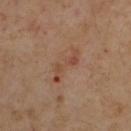lesion size=~5 mm (longest diameter) | automated lesion analysis=a lesion area of about 5.5 mm², an eccentricity of roughly 0.95, and a symmetry-axis asymmetry near 0.5; a border-irregularity rating of about 8/10, internal color variation of about 4 on a 0–10 scale, and peripheral color asymmetry of about 1 | illumination=cross-polarized illumination | image=~15 mm crop, total-body skin-cancer survey | anatomic site=the left upper arm | patient=male, in their mid- to late 50s.